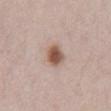Background:
The lesion is located on the abdomen. The lesion's longest dimension is about 3 mm. The subject is a male roughly 70 years of age. A roughly 15 mm field-of-view crop from a total-body skin photograph.